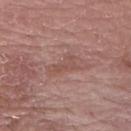Recorded during total-body skin imaging; not selected for excision or biopsy. Located on the right forearm. This is a white-light tile. A male subject, in their 60s. About 3 mm across. The total-body-photography lesion software estimated a lesion color around L≈50 a*≈21 b*≈24 in CIELAB, roughly 7 lightness units darker than nearby skin, and a normalized lesion–skin contrast near 5. This image is a 15 mm lesion crop taken from a total-body photograph.